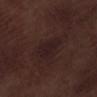The lesion was tiled from a total-body skin photograph and was not biopsied. About 4 mm across. Automated tile analysis of the lesion measured an area of roughly 7.5 mm², an eccentricity of roughly 0.8, and a symmetry-axis asymmetry near 0.3. And it measured a normalized lesion–skin contrast near 7. It also reported internal color variation of about 1.5 on a 0–10 scale. A lesion tile, about 15 mm wide, cut from a 3D total-body photograph. A male patient, in their 70s. Located on the left lower leg.